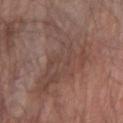Impression: Captured during whole-body skin photography for melanoma surveillance; the lesion was not biopsied.